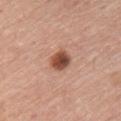No biopsy was performed on this lesion — it was imaged during a full skin examination and was not determined to be concerning.
On the chest.
A lesion tile, about 15 mm wide, cut from a 3D total-body photograph.
A male subject, aged 58 to 62.